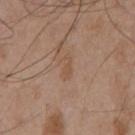biopsy status: catalogued during a skin exam; not biopsied
automated lesion analysis: an area of roughly 2.5 mm², an eccentricity of roughly 0.95, and a symmetry-axis asymmetry near 0.5; a mean CIELAB color near L≈51 a*≈18 b*≈30; a border-irregularity rating of about 5.5/10, a color-variation rating of about 0/10, and a peripheral color-asymmetry measure near 0; an automated nevus-likeness rating near 0 out of 100 and a lesion-detection confidence of about 100/100
tile lighting: white-light
anatomic site: the chest
size: ~3 mm (longest diameter)
patient: male, about 55 years old
imaging modality: ~15 mm crop, total-body skin-cancer survey